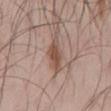This lesion was catalogued during total-body skin photography and was not selected for biopsy. Captured under white-light illumination. From the mid back. A close-up tile cropped from a whole-body skin photograph, about 15 mm across. The patient is a male approximately 45 years of age. The recorded lesion diameter is about 4.5 mm.